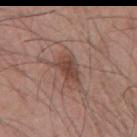follow-up = imaged on a skin check; not biopsied.